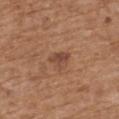Impression:
Captured during whole-body skin photography for melanoma surveillance; the lesion was not biopsied.
Context:
Located on the upper back. The recorded lesion diameter is about 2.5 mm. Automated tile analysis of the lesion measured a lesion color around L≈45 a*≈21 b*≈29 in CIELAB. And it measured border irregularity of about 3.5 on a 0–10 scale, a within-lesion color-variation index near 2/10, and radial color variation of about 0.5. A region of skin cropped from a whole-body photographic capture, roughly 15 mm wide. The patient is a male aged around 70. Captured under white-light illumination.